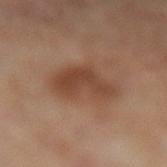No biopsy was performed on this lesion — it was imaged during a full skin examination and was not determined to be concerning.
A female patient aged approximately 60.
From the left lower leg.
A 15 mm crop from a total-body photograph taken for skin-cancer surveillance.